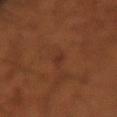follow-up — imaged on a skin check; not biopsied
site — the right forearm
illumination — cross-polarized illumination
subject — male, aged around 55
lesion size — ≈3.5 mm
image — total-body-photography crop, ~15 mm field of view